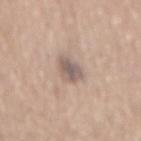patient: male, approximately 65 years of age
site: the mid back
acquisition: ~15 mm crop, total-body skin-cancer survey